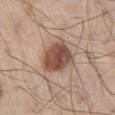Clinical impression: This lesion was catalogued during total-body skin photography and was not selected for biopsy. Clinical summary: The patient is a male roughly 60 years of age. Captured under white-light illumination. Automated tile analysis of the lesion measured a lesion–skin lightness drop of about 15 and a normalized lesion–skin contrast near 10.5. And it measured a border-irregularity rating of about 2/10 and internal color variation of about 5 on a 0–10 scale. The analysis additionally found an automated nevus-likeness rating near 95 out of 100. The lesion is on the right thigh. Approximately 4.5 mm at its widest. A close-up tile cropped from a whole-body skin photograph, about 15 mm across.